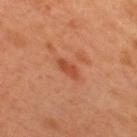This lesion was catalogued during total-body skin photography and was not selected for biopsy. The subject is a male aged 48 to 52. On the upper back. A close-up tile cropped from a whole-body skin photograph, about 15 mm across. Captured under cross-polarized illumination.